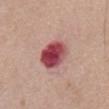Clinical impression: Captured during whole-body skin photography for melanoma surveillance; the lesion was not biopsied. Clinical summary: The subject is a male roughly 70 years of age. Captured under white-light illumination. From the chest. A 15 mm close-up tile from a total-body photography series done for melanoma screening. Longest diameter approximately 4.5 mm.